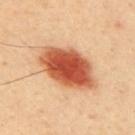image:
  source: total-body photography crop
  field_of_view_mm: 15
patient:
  sex: male
  age_approx: 40
lighting: cross-polarized
automated_metrics:
  color_variation_0_10: 7.0
  peripheral_color_asymmetry: 2.0
site: upper back